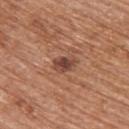image:
  source: total-body photography crop
  field_of_view_mm: 15
patient:
  sex: female
  age_approx: 70
lighting: white-light
lesion_size:
  long_diameter_mm_approx: 3.0
site: upper back